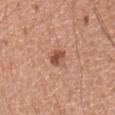notes=no biopsy performed (imaged during a skin exam) | acquisition=~15 mm crop, total-body skin-cancer survey | lighting=white-light illumination | size=≈2.5 mm | site=the right upper arm | patient=male, aged 38–42.